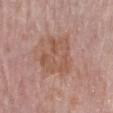The lesion is located on the right forearm.
The patient is a female in their mid- to late 60s.
A roughly 15 mm field-of-view crop from a total-body skin photograph.